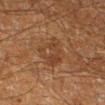<lesion>
  <biopsy_status>not biopsied; imaged during a skin examination</biopsy_status>
  <image>
    <source>total-body photography crop</source>
    <field_of_view_mm>15</field_of_view_mm>
  </image>
  <patient>
    <sex>male</sex>
    <age_approx>60</age_approx>
  </patient>
  <lighting>cross-polarized</lighting>
  <site>right lower leg</site>
</lesion>